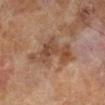Longest diameter approximately 6.5 mm. A male subject aged around 65. The total-body-photography lesion software estimated an area of roughly 14 mm² and a shape-asymmetry score of about 0.6 (0 = symmetric). It also reported a border-irregularity rating of about 7.5/10, a within-lesion color-variation index near 4.5/10, and a peripheral color-asymmetry measure near 1.5. A close-up tile cropped from a whole-body skin photograph, about 15 mm across.